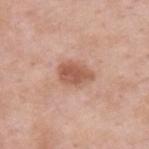Impression: Captured during whole-body skin photography for melanoma surveillance; the lesion was not biopsied. Acquisition and patient details: The patient is a male approximately 55 years of age. Longest diameter approximately 4 mm. A close-up tile cropped from a whole-body skin photograph, about 15 mm across. The lesion is located on the upper back. The lesion-visualizer software estimated an area of roughly 8 mm² and two-axis asymmetry of about 0.2. And it measured an average lesion color of about L≈56 a*≈23 b*≈30 (CIELAB), about 12 CIELAB-L* units darker than the surrounding skin, and a normalized lesion–skin contrast near 8. It also reported a border-irregularity index near 2/10, a within-lesion color-variation index near 2.5/10, and a peripheral color-asymmetry measure near 1. It also reported a nevus-likeness score of about 55/100 and a lesion-detection confidence of about 100/100. Imaged with white-light lighting.